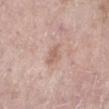Q: Is there a histopathology result?
A: no biopsy performed (imaged during a skin exam)
Q: Lesion location?
A: the leg
Q: What did automated image analysis measure?
A: roughly 8 lightness units darker than nearby skin and a normalized border contrast of about 5.5; peripheral color asymmetry of about 0.5; a classifier nevus-likeness of about 0/100 and lesion-presence confidence of about 100/100
Q: What lighting was used for the tile?
A: white-light illumination
Q: Patient demographics?
A: female, approximately 70 years of age
Q: What is the imaging modality?
A: ~15 mm crop, total-body skin-cancer survey